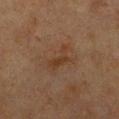Clinical impression: The lesion was photographed on a routine skin check and not biopsied; there is no pathology result. Background: The subject is a female about 55 years old. From the chest. The total-body-photography lesion software estimated a footprint of about 5 mm², an eccentricity of roughly 0.85, and a symmetry-axis asymmetry near 0.4. The software also gave a nevus-likeness score of about 0/100 and a detector confidence of about 100 out of 100 that the crop contains a lesion. A roughly 15 mm field-of-view crop from a total-body skin photograph. The recorded lesion diameter is about 3.5 mm. Imaged with cross-polarized lighting.